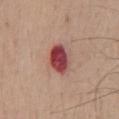Assessment:
This lesion was catalogued during total-body skin photography and was not selected for biopsy.
Clinical summary:
Cropped from a whole-body photographic skin survey; the tile spans about 15 mm. The recorded lesion diameter is about 4 mm. This is a white-light tile. A male subject, in their mid- to late 70s. The lesion is located on the chest.